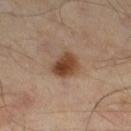Q: Was a biopsy performed?
A: catalogued during a skin exam; not biopsied
Q: Who is the patient?
A: male, in their mid-40s
Q: Lesion size?
A: about 3.5 mm
Q: Illumination type?
A: cross-polarized illumination
Q: What kind of image is this?
A: ~15 mm crop, total-body skin-cancer survey
Q: Where on the body is the lesion?
A: the right thigh
Q: Automated lesion metrics?
A: a lesion-to-skin contrast of about 10.5 (normalized; higher = more distinct); a border-irregularity index near 2/10 and a within-lesion color-variation index near 5/10